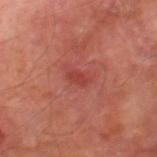follow-up — imaged on a skin check; not biopsied
size — about 2.5 mm
patient — male, aged 68–72
location — the right thigh
image source — ~15 mm tile from a whole-body skin photo